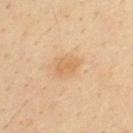  biopsy_status: not biopsied; imaged during a skin examination
  lighting: cross-polarized
  patient:
    sex: male
    age_approx: 35
  lesion_size:
    long_diameter_mm_approx: 3.0
  image:
    source: total-body photography crop
    field_of_view_mm: 15
  site: upper back